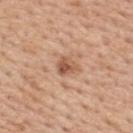Findings:
– follow-up — catalogued during a skin exam; not biopsied
– patient — female, in their 50s
– location — the back
– size — ~2.5 mm (longest diameter)
– TBP lesion metrics — a footprint of about 4.5 mm², an eccentricity of roughly 0.5, and two-axis asymmetry of about 0.3; a mean CIELAB color near L≈56 a*≈22 b*≈32 and roughly 11 lightness units darker than nearby skin; a within-lesion color-variation index near 4.5/10 and radial color variation of about 1.5
– tile lighting — white-light
– image source — ~15 mm crop, total-body skin-cancer survey